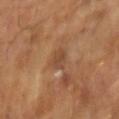workup = imaged on a skin check; not biopsied | illumination = cross-polarized illumination | lesion size = ~2.5 mm (longest diameter) | acquisition = total-body-photography crop, ~15 mm field of view | patient = male, aged around 65.